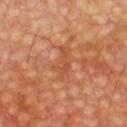No biopsy was performed on this lesion — it was imaged during a full skin examination and was not determined to be concerning. Located on the chest. A male subject, approximately 60 years of age. About 4.5 mm across. Automated tile analysis of the lesion measured a footprint of about 5.5 mm², a shape eccentricity near 0.9, and a symmetry-axis asymmetry near 0.5. And it measured an automated nevus-likeness rating near 0 out of 100 and a lesion-detection confidence of about 90/100. Imaged with cross-polarized lighting. Cropped from a whole-body photographic skin survey; the tile spans about 15 mm.